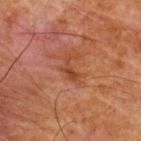biopsy status: imaged on a skin check; not biopsied | location: the back | automated metrics: an average lesion color of about L≈35 a*≈23 b*≈29 (CIELAB), about 6 CIELAB-L* units darker than the surrounding skin, and a lesion-to-skin contrast of about 6.5 (normalized; higher = more distinct); a peripheral color-asymmetry measure near 0; an automated nevus-likeness rating near 0 out of 100 and lesion-presence confidence of about 100/100 | lesion diameter: ~3 mm (longest diameter) | patient: male, aged around 65 | acquisition: 15 mm crop, total-body photography.